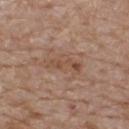Clinical impression:
The lesion was photographed on a routine skin check and not biopsied; there is no pathology result.
Context:
Measured at roughly 4 mm in maximum diameter. A 15 mm close-up extracted from a 3D total-body photography capture. The lesion is on the upper back. A male subject about 80 years old.